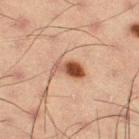Part of a total-body skin-imaging series; this lesion was reviewed on a skin check and was not flagged for biopsy. The lesion's longest dimension is about 3.5 mm. Captured under cross-polarized illumination. From the left thigh. A male patient, aged 53 to 57. A region of skin cropped from a whole-body photographic capture, roughly 15 mm wide.